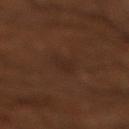{"biopsy_status": "not biopsied; imaged during a skin examination", "lesion_size": {"long_diameter_mm_approx": 3.5}, "site": "right lower leg", "patient": {"sex": "male", "age_approx": 65}, "image": {"source": "total-body photography crop", "field_of_view_mm": 15}}